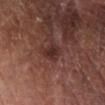Assessment: The lesion was tiled from a total-body skin photograph and was not biopsied. Acquisition and patient details: A male subject, in their 70s. The lesion is on the right lower leg. Cropped from a total-body skin-imaging series; the visible field is about 15 mm.